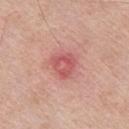Impression:
Captured during whole-body skin photography for melanoma surveillance; the lesion was not biopsied.
Image and clinical context:
About 3 mm across. The tile uses white-light illumination. The lesion is on the chest. A 15 mm crop from a total-body photograph taken for skin-cancer surveillance. An algorithmic analysis of the crop reported a lesion color around L≈57 a*≈32 b*≈25 in CIELAB, about 10 CIELAB-L* units darker than the surrounding skin, and a lesion-to-skin contrast of about 6.5 (normalized; higher = more distinct). The analysis additionally found a nevus-likeness score of about 0/100. The patient is a male about 60 years old.